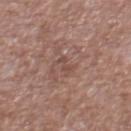  biopsy_status: not biopsied; imaged during a skin examination
  lighting: white-light
  site: abdomen
  image:
    source: total-body photography crop
    field_of_view_mm: 15
  patient:
    sex: male
    age_approx: 65
  lesion_size:
    long_diameter_mm_approx: 2.5
  automated_metrics:
    eccentricity: 0.95
    shape_asymmetry: 0.45
    vs_skin_darker_L: 6.0
    vs_skin_contrast_norm: 5.0